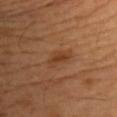On the chest.
A roughly 15 mm field-of-view crop from a total-body skin photograph.
Automated image analysis of the tile measured a border-irregularity index near 4/10 and peripheral color asymmetry of about 0. It also reported a nevus-likeness score of about 5/100 and a lesion-detection confidence of about 100/100.
A male patient aged around 40.